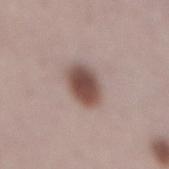biopsy status: catalogued during a skin exam; not biopsied | lesion diameter: ≈4 mm | subject: male, in their 70s | acquisition: ~15 mm tile from a whole-body skin photo | tile lighting: white-light illumination | anatomic site: the mid back | TBP lesion metrics: a lesion–skin lightness drop of about 15 and a lesion-to-skin contrast of about 10.5 (normalized; higher = more distinct); a border-irregularity rating of about 1.5/10, a within-lesion color-variation index near 4.5/10, and a peripheral color-asymmetry measure near 1; a lesion-detection confidence of about 100/100.